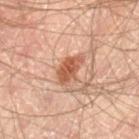Recorded during total-body skin imaging; not selected for excision or biopsy.
Cropped from a whole-body photographic skin survey; the tile spans about 15 mm.
Measured at roughly 4 mm in maximum diameter.
The patient is a male about 65 years old.
On the left thigh.
Imaged with cross-polarized lighting.
An algorithmic analysis of the crop reported roughly 12 lightness units darker than nearby skin and a normalized lesion–skin contrast near 8.